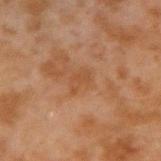Imaged during a routine full-body skin examination; the lesion was not biopsied and no histopathology is available.
The lesion's longest dimension is about 2.5 mm.
A male patient approximately 45 years of age.
Located on the right upper arm.
The lesion-visualizer software estimated roughly 5 lightness units darker than nearby skin. The software also gave a border-irregularity index near 5/10 and a peripheral color-asymmetry measure near 0.5.
A roughly 15 mm field-of-view crop from a total-body skin photograph.
The tile uses cross-polarized illumination.